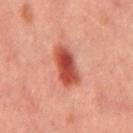Q: Is there a histopathology result?
A: catalogued during a skin exam; not biopsied
Q: How was this image acquired?
A: ~15 mm tile from a whole-body skin photo
Q: What is the anatomic site?
A: the mid back
Q: What are the patient's age and sex?
A: male, approximately 45 years of age